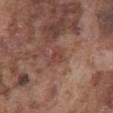The lesion was photographed on a routine skin check and not biopsied; there is no pathology result. An algorithmic analysis of the crop reported a mean CIELAB color near L≈43 a*≈20 b*≈25, a lesion–skin lightness drop of about 7, and a normalized lesion–skin contrast near 5.5. It also reported a classifier nevus-likeness of about 0/100 and a lesion-detection confidence of about 100/100. A male patient, aged around 75. The lesion is on the abdomen. Imaged with white-light lighting. A roughly 15 mm field-of-view crop from a total-body skin photograph. The lesion's longest dimension is about 2.5 mm.